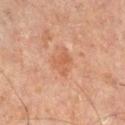Assessment:
The lesion was tiled from a total-body skin photograph and was not biopsied.
Context:
The subject is a male roughly 65 years of age. Located on the right lower leg. Cropped from a whole-body photographic skin survey; the tile spans about 15 mm.